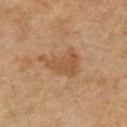No biopsy was performed on this lesion — it was imaged during a full skin examination and was not determined to be concerning. Measured at roughly 5 mm in maximum diameter. The subject is a female approximately 60 years of age. Automated tile analysis of the lesion measured a mean CIELAB color near L≈45 a*≈17 b*≈31. The analysis additionally found an automated nevus-likeness rating near 30 out of 100 and a lesion-detection confidence of about 100/100. From the left upper arm. This is a cross-polarized tile. A 15 mm crop from a total-body photograph taken for skin-cancer surveillance.